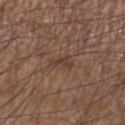| feature | finding |
|---|---|
| follow-up | catalogued during a skin exam; not biopsied |
| patient | male, in their mid-50s |
| image | ~15 mm tile from a whole-body skin photo |
| illumination | white-light illumination |
| location | the right lower leg |